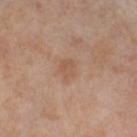biopsy status — catalogued during a skin exam; not biopsied
subject — female, approximately 55 years of age
imaging modality — 15 mm crop, total-body photography
tile lighting — cross-polarized illumination
location — the left thigh
TBP lesion metrics — a classifier nevus-likeness of about 0/100
size — ≈3 mm